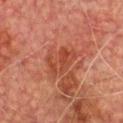notes: total-body-photography surveillance lesion; no biopsy | body site: the chest | automated metrics: an average lesion color of about L≈38 a*≈26 b*≈29 (CIELAB), roughly 7 lightness units darker than nearby skin, and a lesion-to-skin contrast of about 6 (normalized; higher = more distinct); a border-irregularity index near 6.5/10; a nevus-likeness score of about 0/100 and a lesion-detection confidence of about 100/100 | patient: male, aged 73–77 | tile lighting: cross-polarized illumination | image source: ~15 mm tile from a whole-body skin photo.